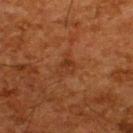| feature | finding |
|---|---|
| biopsy status | imaged on a skin check; not biopsied |
| image source | ~15 mm crop, total-body skin-cancer survey |
| location | the upper back |
| subject | male, aged 63–67 |